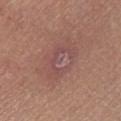Recorded during total-body skin imaging; not selected for excision or biopsy.
A 15 mm crop from a total-body photograph taken for skin-cancer surveillance.
The lesion is on the left lower leg.
Measured at roughly 5 mm in maximum diameter.
The lesion-visualizer software estimated internal color variation of about 5 on a 0–10 scale and peripheral color asymmetry of about 1.5. And it measured a classifier nevus-likeness of about 0/100.
A female patient, approximately 45 years of age.
The tile uses white-light illumination.